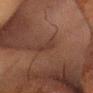Recorded during total-body skin imaging; not selected for excision or biopsy. A male subject aged around 65. A region of skin cropped from a whole-body photographic capture, roughly 15 mm wide. From the head or neck. An algorithmic analysis of the crop reported a shape eccentricity near 0.85 and a symmetry-axis asymmetry near 0.25. It also reported an average lesion color of about L≈29 a*≈16 b*≈21 (CIELAB), about 5 CIELAB-L* units darker than the surrounding skin, and a normalized lesion–skin contrast near 5. The analysis additionally found a nevus-likeness score of about 0/100 and a detector confidence of about 65 out of 100 that the crop contains a lesion. Captured under cross-polarized illumination.